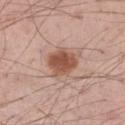Part of a total-body skin-imaging series; this lesion was reviewed on a skin check and was not flagged for biopsy.
Longest diameter approximately 4 mm.
This image is a 15 mm lesion crop taken from a total-body photograph.
A male patient approximately 55 years of age.
The lesion is located on the right thigh.